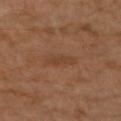follow-up — no biopsy performed (imaged during a skin exam)
lighting — cross-polarized
image source — ~15 mm crop, total-body skin-cancer survey
patient — female, approximately 70 years of age
lesion size — ~4 mm (longest diameter)
body site — the left forearm